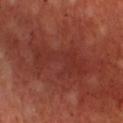{
  "biopsy_status": "not biopsied; imaged during a skin examination",
  "lesion_size": {
    "long_diameter_mm_approx": 7.5
  },
  "patient": {
    "sex": "male",
    "age_approx": 55
  },
  "lighting": "cross-polarized",
  "image": {
    "source": "total-body photography crop",
    "field_of_view_mm": 15
  },
  "site": "chest"
}